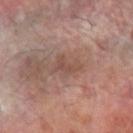{
  "biopsy_status": "not biopsied; imaged during a skin examination",
  "lesion_size": {
    "long_diameter_mm_approx": 3.5
  },
  "site": "left forearm",
  "patient": {
    "sex": "male",
    "age_approx": 70
  },
  "image": {
    "source": "total-body photography crop",
    "field_of_view_mm": 15
  },
  "lighting": "cross-polarized"
}